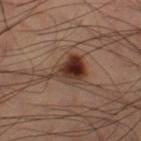The lesion was tiled from a total-body skin photograph and was not biopsied. A close-up tile cropped from a whole-body skin photograph, about 15 mm across. Automated image analysis of the tile measured a lesion area of about 10 mm² and a symmetry-axis asymmetry near 0.4. And it measured a lesion color around L≈34 a*≈19 b*≈25 in CIELAB, about 14 CIELAB-L* units darker than the surrounding skin, and a lesion-to-skin contrast of about 12 (normalized; higher = more distinct). The software also gave internal color variation of about 10 on a 0–10 scale and a peripheral color-asymmetry measure near 4. It also reported a nevus-likeness score of about 100/100. The lesion is on the left thigh. A male subject aged around 60. Approximately 5 mm at its widest.